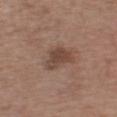follow-up: total-body-photography surveillance lesion; no biopsy
subject: male, aged 53 to 57
image source: total-body-photography crop, ~15 mm field of view
lighting: white-light illumination
TBP lesion metrics: a border-irregularity index near 4/10 and a within-lesion color-variation index near 3/10; an automated nevus-likeness rating near 35 out of 100 and lesion-presence confidence of about 100/100
anatomic site: the left upper arm
lesion size: about 4 mm